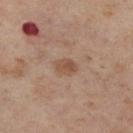Captured during whole-body skin photography for melanoma surveillance; the lesion was not biopsied. The subject is a female in their mid- to late 50s. About 3 mm across. A 15 mm close-up extracted from a 3D total-body photography capture. From the right lower leg. Captured under cross-polarized illumination.